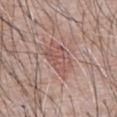Background: The lesion is on the abdomen. A male subject, aged around 60. A 15 mm crop from a total-body photograph taken for skin-cancer surveillance. Approximately 4.5 mm at its widest. Captured under white-light illumination.